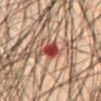{
  "biopsy_status": "not biopsied; imaged during a skin examination",
  "automated_metrics": {
    "nevus_likeness_0_100": 0,
    "lesion_detection_confidence_0_100": 100
  },
  "lighting": "cross-polarized",
  "lesion_size": {
    "long_diameter_mm_approx": 4.0
  },
  "image": {
    "source": "total-body photography crop",
    "field_of_view_mm": 15
  },
  "patient": {
    "sex": "male",
    "age_approx": 45
  },
  "site": "front of the torso"
}